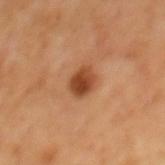{"site": "mid back", "lesion_size": {"long_diameter_mm_approx": 3.0}, "image": {"source": "total-body photography crop", "field_of_view_mm": 15}, "patient": {"sex": "male", "age_approx": 65}}